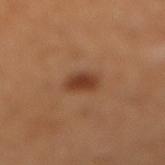• notes — total-body-photography surveillance lesion; no biopsy
• site — the right lower leg
• subject — female, aged approximately 55
• image — ~15 mm tile from a whole-body skin photo